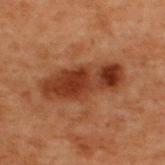Part of a total-body skin-imaging series; this lesion was reviewed on a skin check and was not flagged for biopsy. From the back. A lesion tile, about 15 mm wide, cut from a 3D total-body photograph. An algorithmic analysis of the crop reported a symmetry-axis asymmetry near 0.15. And it measured a lesion color around L≈29 a*≈21 b*≈27 in CIELAB, roughly 10 lightness units darker than nearby skin, and a lesion-to-skin contrast of about 10 (normalized; higher = more distinct). The analysis additionally found border irregularity of about 2.5 on a 0–10 scale, internal color variation of about 6 on a 0–10 scale, and a peripheral color-asymmetry measure near 2. The recorded lesion diameter is about 8 mm. This is a cross-polarized tile. A male patient roughly 70 years of age.